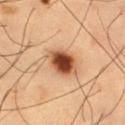notes = total-body-photography surveillance lesion; no biopsy
tile lighting = cross-polarized
image source = 15 mm crop, total-body photography
subject = male, aged around 60
location = the left thigh
lesion size = about 3.5 mm